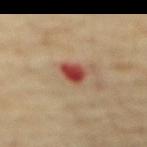The lesion was tiled from a total-body skin photograph and was not biopsied. The recorded lesion diameter is about 3 mm. Cropped from a total-body skin-imaging series; the visible field is about 15 mm. Captured under cross-polarized illumination. From the abdomen. The patient is a male roughly 65 years of age.